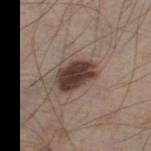| field | value |
|---|---|
| follow-up | catalogued during a skin exam; not biopsied |
| lighting | white-light illumination |
| diameter | about 4.5 mm |
| location | the right thigh |
| subject | male, in their 60s |
| imaging modality | ~15 mm tile from a whole-body skin photo |